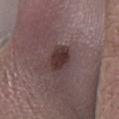Impression:
This lesion was catalogued during total-body skin photography and was not selected for biopsy.
Background:
On the right lower leg. Automated tile analysis of the lesion measured peripheral color asymmetry of about 1. The analysis additionally found a lesion-detection confidence of about 100/100. A 15 mm crop from a total-body photograph taken for skin-cancer surveillance. The tile uses white-light illumination. Approximately 3.5 mm at its widest. A male subject, aged 43 to 47.